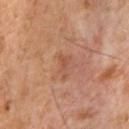Assessment: This lesion was catalogued during total-body skin photography and was not selected for biopsy. Background: About 3 mm across. The total-body-photography lesion software estimated a footprint of about 3 mm², an outline eccentricity of about 0.9 (0 = round, 1 = elongated), and two-axis asymmetry of about 0.6. And it measured internal color variation of about 0.5 on a 0–10 scale and a peripheral color-asymmetry measure near 0. A male patient, aged approximately 60. A 15 mm close-up tile from a total-body photography series done for melanoma screening. The lesion is on the chest.